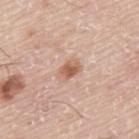biopsy status = imaged on a skin check; not biopsied | acquisition = total-body-photography crop, ~15 mm field of view | subject = male, about 75 years old | TBP lesion metrics = a mean CIELAB color near L≈59 a*≈22 b*≈31 and a lesion–skin lightness drop of about 12 | tile lighting = white-light | site = the back | lesion diameter = ~2.5 mm (longest diameter).